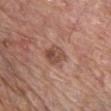On the chest.
Automated image analysis of the tile measured roughly 10 lightness units darker than nearby skin and a normalized border contrast of about 7.5. It also reported a border-irregularity rating of about 1.5/10, internal color variation of about 5 on a 0–10 scale, and peripheral color asymmetry of about 2. It also reported lesion-presence confidence of about 100/100.
A 15 mm close-up tile from a total-body photography series done for melanoma screening.
A male subject roughly 65 years of age.
About 2.5 mm across.
This is a white-light tile.